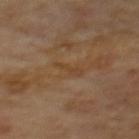The lesion was tiled from a total-body skin photograph and was not biopsied.
A roughly 15 mm field-of-view crop from a total-body skin photograph.
An algorithmic analysis of the crop reported an area of roughly 3 mm², a shape eccentricity near 0.95, and a shape-asymmetry score of about 0.35 (0 = symmetric). The analysis additionally found a nevus-likeness score of about 0/100 and a lesion-detection confidence of about 95/100.
On the mid back.
Imaged with cross-polarized lighting.
Measured at roughly 3 mm in maximum diameter.
The patient is a male roughly 70 years of age.